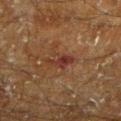The lesion was photographed on a routine skin check and not biopsied; there is no pathology result.
This is a cross-polarized tile.
A region of skin cropped from a whole-body photographic capture, roughly 15 mm wide.
About 3.5 mm across.
Located on the left lower leg.
A male patient, about 60 years old.